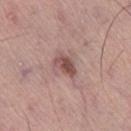<tbp_lesion>
  <biopsy_status>not biopsied; imaged during a skin examination</biopsy_status>
  <image>
    <source>total-body photography crop</source>
    <field_of_view_mm>15</field_of_view_mm>
  </image>
  <automated_metrics>
    <border_irregularity_0_10>2.0</border_irregularity_0_10>
    <color_variation_0_10>3.5</color_variation_0_10>
    <peripheral_color_asymmetry>1.5</peripheral_color_asymmetry>
    <nevus_likeness_0_100>70</nevus_likeness_0_100>
    <lesion_detection_confidence_0_100>100</lesion_detection_confidence_0_100>
  </automated_metrics>
  <lesion_size>
    <long_diameter_mm_approx>3.0</long_diameter_mm_approx>
  </lesion_size>
  <lighting>white-light</lighting>
  <site>right lower leg</site>
  <patient>
    <sex>male</sex>
    <age_approx>55</age_approx>
  </patient>
</tbp_lesion>